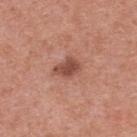Findings:
- biopsy status: imaged on a skin check; not biopsied
- site: the upper back
- patient: female, roughly 35 years of age
- image: ~15 mm crop, total-body skin-cancer survey